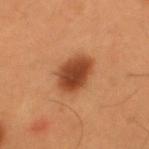workup: imaged on a skin check; not biopsied | automated lesion analysis: a footprint of about 11 mm², an outline eccentricity of about 0.7 (0 = round, 1 = elongated), and a symmetry-axis asymmetry near 0.15; a classifier nevus-likeness of about 100/100 | body site: the upper back | imaging modality: total-body-photography crop, ~15 mm field of view | subject: male, about 55 years old | size: ≈4.5 mm.